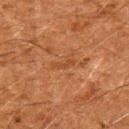| key | value |
|---|---|
| workup | total-body-photography surveillance lesion; no biopsy |
| image source | 15 mm crop, total-body photography |
| size | ≈2.5 mm |
| subject | male, approximately 60 years of age |
| illumination | cross-polarized illumination |
| location | the left upper arm |